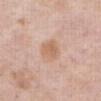Q: Was a biopsy performed?
A: imaged on a skin check; not biopsied
Q: Automated lesion metrics?
A: a footprint of about 7 mm², a shape eccentricity near 0.35, and a symmetry-axis asymmetry near 0.25; a lesion–skin lightness drop of about 8 and a normalized border contrast of about 6.5; a border-irregularity index near 2.5/10 and a color-variation rating of about 2.5/10
Q: Who is the patient?
A: female, aged 63–67
Q: Where on the body is the lesion?
A: the front of the torso
Q: Illumination type?
A: white-light
Q: What is the imaging modality?
A: 15 mm crop, total-body photography
Q: How large is the lesion?
A: about 3 mm